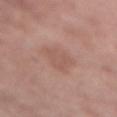| feature | finding |
|---|---|
| notes | total-body-photography surveillance lesion; no biopsy |
| automated metrics | a lesion area of about 10 mm², an outline eccentricity of about 0.65 (0 = round, 1 = elongated), and a symmetry-axis asymmetry near 0.3; a mean CIELAB color near L≈55 a*≈20 b*≈25, about 6 CIELAB-L* units darker than the surrounding skin, and a normalized border contrast of about 4.5; a border-irregularity index near 3.5/10, internal color variation of about 2 on a 0–10 scale, and radial color variation of about 0.5; an automated nevus-likeness rating near 0 out of 100 and lesion-presence confidence of about 100/100 |
| illumination | white-light illumination |
| image source | ~15 mm tile from a whole-body skin photo |
| location | the chest |
| subject | female, roughly 75 years of age |